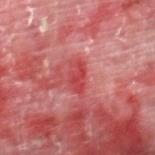Clinical impression:
Imaged during a routine full-body skin examination; the lesion was not biopsied and no histopathology is available.
Background:
This is a cross-polarized tile. A lesion tile, about 15 mm wide, cut from a 3D total-body photograph. Approximately 3.5 mm at its widest. The lesion is on the right thigh. The patient is a male in their mid-50s.